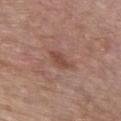Imaged during a routine full-body skin examination; the lesion was not biopsied and no histopathology is available.
Captured under white-light illumination.
A male subject, aged approximately 55.
On the front of the torso.
A lesion tile, about 15 mm wide, cut from a 3D total-body photograph.
Measured at roughly 3.5 mm in maximum diameter.